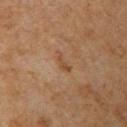On the arm. Longest diameter approximately 2.5 mm. The tile uses cross-polarized illumination. A 15 mm close-up extracted from a 3D total-body photography capture. The patient is a male approximately 60 years of age.